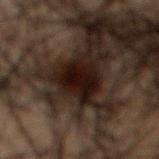Notes:
- workup: total-body-photography surveillance lesion; no biopsy
- location: the mid back
- lesion size: ≈5.5 mm
- tile lighting: cross-polarized
- subject: male, roughly 50 years of age
- automated lesion analysis: a lesion area of about 13 mm² and a shape-asymmetry score of about 0.25 (0 = symmetric); an average lesion color of about L≈10 a*≈11 b*≈11 (CIELAB), about 10 CIELAB-L* units darker than the surrounding skin, and a normalized border contrast of about 15; a detector confidence of about 100 out of 100 that the crop contains a lesion
- acquisition: 15 mm crop, total-body photography